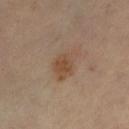biopsy_status: not biopsied; imaged during a skin examination
site: left lower leg
image:
  source: total-body photography crop
  field_of_view_mm: 15
patient:
  sex: female
  age_approx: 60